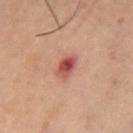<record>
  <biopsy_status>not biopsied; imaged during a skin examination</biopsy_status>
  <lesion_size>
    <long_diameter_mm_approx>3.0</long_diameter_mm_approx>
  </lesion_size>
  <site>abdomen</site>
  <image>
    <source>total-body photography crop</source>
    <field_of_view_mm>15</field_of_view_mm>
  </image>
  <lighting>cross-polarized</lighting>
  <patient>
    <sex>female</sex>
    <age_approx>55</age_approx>
  </patient>
</record>